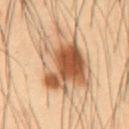Findings:
* body site: the abdomen
* image source: total-body-photography crop, ~15 mm field of view
* lighting: cross-polarized
* lesion diameter: ≈7.5 mm
* subject: male, aged 53 to 57